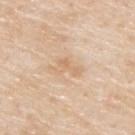Automated image analysis of the tile measured a footprint of about 4 mm² and two-axis asymmetry of about 0.55. And it measured a border-irregularity rating of about 6.5/10 and a peripheral color-asymmetry measure near 0.5.
A 15 mm close-up extracted from a 3D total-body photography capture.
Captured under white-light illumination.
The lesion is located on the back.
A male subject, approximately 80 years of age.
Measured at roughly 3.5 mm in maximum diameter.